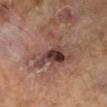workup: total-body-photography surveillance lesion; no biopsy
tile lighting: cross-polarized
image source: ~15 mm crop, total-body skin-cancer survey
lesion diameter: ≈6 mm
patient: male, aged around 65